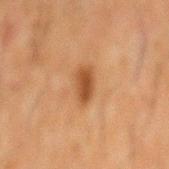Part of a total-body skin-imaging series; this lesion was reviewed on a skin check and was not flagged for biopsy. The patient is a male in their 60s. A lesion tile, about 15 mm wide, cut from a 3D total-body photograph. The total-body-photography lesion software estimated border irregularity of about 3 on a 0–10 scale, internal color variation of about 2 on a 0–10 scale, and a peripheral color-asymmetry measure near 0.5. The recorded lesion diameter is about 3.5 mm. From the mid back. Imaged with cross-polarized lighting.